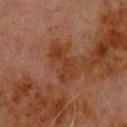  biopsy_status: not biopsied; imaged during a skin examination
  image:
    source: total-body photography crop
    field_of_view_mm: 15
  lighting: cross-polarized
  automated_metrics:
    cielab_L: 28
    cielab_a: 19
    cielab_b: 27
    vs_skin_darker_L: 6.0
    vs_skin_contrast_norm: 7.0
    border_irregularity_0_10: 5.5
    peripheral_color_asymmetry: 0.5
  site: upper back
  patient:
    sex: male
    age_approx: 80